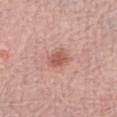No biopsy was performed on this lesion — it was imaged during a full skin examination and was not determined to be concerning. The lesion's longest dimension is about 2.5 mm. A 15 mm close-up tile from a total-body photography series done for melanoma screening. A female patient roughly 65 years of age. Automated tile analysis of the lesion measured a lesion area of about 4.5 mm² and a shape-asymmetry score of about 0.3 (0 = symmetric). The analysis additionally found a mean CIELAB color near L≈56 a*≈24 b*≈27. The software also gave a border-irregularity rating of about 2.5/10, internal color variation of about 1.5 on a 0–10 scale, and radial color variation of about 0.5. From the right forearm.